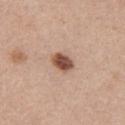A female patient about 45 years old. From the right upper arm. This image is a 15 mm lesion crop taken from a total-body photograph. The lesion-visualizer software estimated border irregularity of about 1.5 on a 0–10 scale, a within-lesion color-variation index near 3.5/10, and radial color variation of about 1. The software also gave a classifier nevus-likeness of about 95/100 and a detector confidence of about 100 out of 100 that the crop contains a lesion. The lesion's longest dimension is about 3 mm. Imaged with white-light lighting.